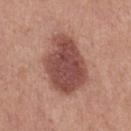biopsy_status: not biopsied; imaged during a skin examination
image:
  source: total-body photography crop
  field_of_view_mm: 15
site: left thigh
lesion_size:
  long_diameter_mm_approx: 7.0
patient:
  sex: female
  age_approx: 40